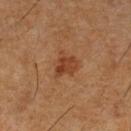Case summary:
• notes — no biopsy performed (imaged during a skin exam)
• lighting — cross-polarized illumination
• subject — male, roughly 60 years of age
• image source — total-body-photography crop, ~15 mm field of view
• body site — the left lower leg